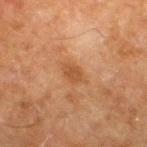The lesion was photographed on a routine skin check and not biopsied; there is no pathology result. The total-body-photography lesion software estimated an area of roughly 4.5 mm², an outline eccentricity of about 0.8 (0 = round, 1 = elongated), and two-axis asymmetry of about 0.25. It also reported a border-irregularity index near 2.5/10 and peripheral color asymmetry of about 0.5. A male patient aged approximately 80. The tile uses cross-polarized illumination. On the leg. Cropped from a whole-body photographic skin survey; the tile spans about 15 mm.